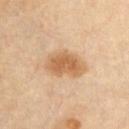Q: Was this lesion biopsied?
A: catalogued during a skin exam; not biopsied
Q: What lighting was used for the tile?
A: cross-polarized illumination
Q: Patient demographics?
A: male, aged 63 to 67
Q: What did automated image analysis measure?
A: a mean CIELAB color near L≈59 a*≈18 b*≈36, about 11 CIELAB-L* units darker than the surrounding skin, and a lesion-to-skin contrast of about 8 (normalized; higher = more distinct); a border-irregularity rating of about 2/10 and radial color variation of about 1
Q: How large is the lesion?
A: ≈5 mm
Q: What is the imaging modality?
A: ~15 mm crop, total-body skin-cancer survey
Q: Where on the body is the lesion?
A: the chest